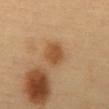Findings:
* workup — total-body-photography surveillance lesion; no biopsy
* location — the front of the torso
* patient — female, aged 58 to 62
* imaging modality — ~15 mm tile from a whole-body skin photo